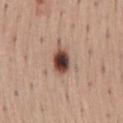Captured during whole-body skin photography for melanoma surveillance; the lesion was not biopsied. A male subject, in their mid-40s. A roughly 15 mm field-of-view crop from a total-body skin photograph. An algorithmic analysis of the crop reported a footprint of about 7 mm², an outline eccentricity of about 0.75 (0 = round, 1 = elongated), and a shape-asymmetry score of about 0.1 (0 = symmetric). It also reported a lesion color around L≈45 a*≈20 b*≈24 in CIELAB, roughly 19 lightness units darker than nearby skin, and a normalized lesion–skin contrast near 13.5. And it measured border irregularity of about 1.5 on a 0–10 scale, internal color variation of about 8.5 on a 0–10 scale, and a peripheral color-asymmetry measure near 2.5. It also reported a nevus-likeness score of about 100/100 and lesion-presence confidence of about 100/100. Located on the lower back. Imaged with white-light lighting.